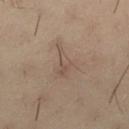Q: Was a biopsy performed?
A: catalogued during a skin exam; not biopsied
Q: Lesion location?
A: the left thigh
Q: How was the tile lit?
A: cross-polarized
Q: What are the patient's age and sex?
A: male, in their 30s
Q: What is the imaging modality?
A: 15 mm crop, total-body photography
Q: Lesion size?
A: ≈4.5 mm